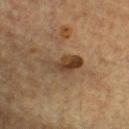Impression: Imaged during a routine full-body skin examination; the lesion was not biopsied and no histopathology is available. Clinical summary: This is a cross-polarized tile. A male patient aged 63 to 67. A region of skin cropped from a whole-body photographic capture, roughly 15 mm wide. Located on the front of the torso.